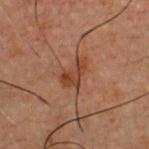Assessment: No biopsy was performed on this lesion — it was imaged during a full skin examination and was not determined to be concerning. Background: Approximately 3.5 mm at its widest. A 15 mm crop from a total-body photograph taken for skin-cancer surveillance. Located on the chest. A male subject in their 60s. An algorithmic analysis of the crop reported border irregularity of about 4.5 on a 0–10 scale and a peripheral color-asymmetry measure near 1.